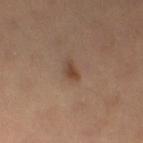| key | value |
|---|---|
| lighting | cross-polarized |
| body site | the leg |
| image | 15 mm crop, total-body photography |
| patient | male, aged 63 to 67 |
| diameter | ~2 mm (longest diameter) |
| automated lesion analysis | a footprint of about 2 mm², an eccentricity of roughly 0.8, and two-axis asymmetry of about 0.4; an average lesion color of about L≈40 a*≈18 b*≈27 (CIELAB), about 9 CIELAB-L* units darker than the surrounding skin, and a normalized lesion–skin contrast near 8; a within-lesion color-variation index near 1/10 and a peripheral color-asymmetry measure near 0.5; a lesion-detection confidence of about 100/100 |